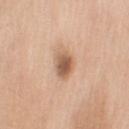* location — the right upper arm
* image source — ~15 mm crop, total-body skin-cancer survey
* size — ~3.5 mm (longest diameter)
* illumination — white-light
* automated metrics — an average lesion color of about L≈59 a*≈20 b*≈32 (CIELAB) and a normalized border contrast of about 8.5; border irregularity of about 2 on a 0–10 scale, a within-lesion color-variation index near 5/10, and peripheral color asymmetry of about 1.5
* subject — female, aged approximately 55